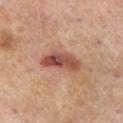Assessment:
No biopsy was performed on this lesion — it was imaged during a full skin examination and was not determined to be concerning.